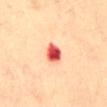This lesion was catalogued during total-body skin photography and was not selected for biopsy.
Cropped from a total-body skin-imaging series; the visible field is about 15 mm.
A female patient aged 38 to 42.
From the back.